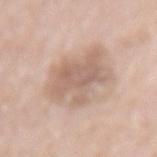The lesion is on the mid back. A female subject in their mid- to late 70s. A 15 mm close-up extracted from a 3D total-body photography capture.